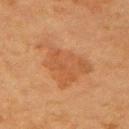The lesion was photographed on a routine skin check and not biopsied; there is no pathology result. Automated image analysis of the tile measured an average lesion color of about L≈44 a*≈21 b*≈33 (CIELAB), about 6 CIELAB-L* units darker than the surrounding skin, and a normalized border contrast of about 5. And it measured a border-irregularity rating of about 6/10, a color-variation rating of about 2.5/10, and peripheral color asymmetry of about 1. A female subject, aged approximately 55. A lesion tile, about 15 mm wide, cut from a 3D total-body photograph. The lesion is on the left upper arm. Measured at roughly 6 mm in maximum diameter.